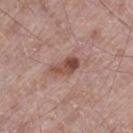Captured during whole-body skin photography for melanoma surveillance; the lesion was not biopsied.
This image is a 15 mm lesion crop taken from a total-body photograph.
The patient is a male roughly 70 years of age.
This is a white-light tile.
The lesion is located on the left thigh.